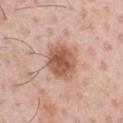No biopsy was performed on this lesion — it was imaged during a full skin examination and was not determined to be concerning. A male patient in their 50s. On the chest. This is a white-light tile. A region of skin cropped from a whole-body photographic capture, roughly 15 mm wide. The lesion's longest dimension is about 4.5 mm.